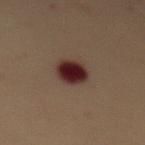Q: Is there a histopathology result?
A: total-body-photography surveillance lesion; no biopsy
Q: How was the tile lit?
A: cross-polarized illumination
Q: What kind of image is this?
A: total-body-photography crop, ~15 mm field of view
Q: How large is the lesion?
A: ≈4 mm
Q: Where on the body is the lesion?
A: the mid back
Q: Patient demographics?
A: female, aged around 50